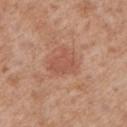<tbp_lesion>
<biopsy_status>not biopsied; imaged during a skin examination</biopsy_status>
<image>
  <source>total-body photography crop</source>
  <field_of_view_mm>15</field_of_view_mm>
</image>
<site>left upper arm</site>
<automated_metrics>
  <vs_skin_darker_L>7.0</vs_skin_darker_L>
  <border_irregularity_0_10>3.0</border_irregularity_0_10>
  <nevus_likeness_0_100>10</nevus_likeness_0_100>
  <lesion_detection_confidence_0_100>100</lesion_detection_confidence_0_100>
</automated_metrics>
<lighting>white-light</lighting>
<patient>
  <sex>male</sex>
  <age_approx>60</age_approx>
</patient>
<lesion_size>
  <long_diameter_mm_approx>3.5</long_diameter_mm_approx>
</lesion_size>
</tbp_lesion>